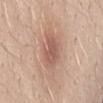{
  "biopsy_status": "not biopsied; imaged during a skin examination",
  "site": "front of the torso",
  "lighting": "white-light",
  "lesion_size": {
    "long_diameter_mm_approx": 3.5
  },
  "image": {
    "source": "total-body photography crop",
    "field_of_view_mm": 15
  },
  "patient": {
    "sex": "female",
    "age_approx": 25
  },
  "automated_metrics": {
    "area_mm2_approx": 7.5,
    "eccentricity": 0.7,
    "cielab_L": 56,
    "cielab_a": 21,
    "cielab_b": 26,
    "vs_skin_contrast_norm": 6.5,
    "border_irregularity_0_10": 3.0,
    "color_variation_0_10": 2.0,
    "peripheral_color_asymmetry": 0.5
  }
}